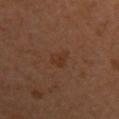{"biopsy_status": "not biopsied; imaged during a skin examination", "lesion_size": {"long_diameter_mm_approx": 3.0}, "image": {"source": "total-body photography crop", "field_of_view_mm": 15}, "site": "left upper arm", "patient": {"sex": "female", "age_approx": 35}, "lighting": "cross-polarized"}